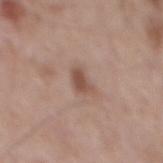lesion diameter=about 2.5 mm
patient=male, roughly 55 years of age
location=the mid back
image=~15 mm crop, total-body skin-cancer survey